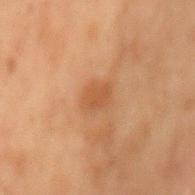| feature | finding |
|---|---|
| workup | catalogued during a skin exam; not biopsied |
| image source | ~15 mm crop, total-body skin-cancer survey |
| site | the lower back |
| subject | male, roughly 70 years of age |